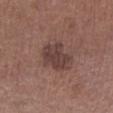Imaged during a routine full-body skin examination; the lesion was not biopsied and no histopathology is available.
Captured under white-light illumination.
This image is a 15 mm lesion crop taken from a total-body photograph.
An algorithmic analysis of the crop reported an area of roughly 10 mm², a shape eccentricity near 0.7, and two-axis asymmetry of about 0.3. The analysis additionally found a lesion color around L≈40 a*≈18 b*≈20 in CIELAB and a normalized border contrast of about 8.
The subject is a male aged 73 to 77.
Located on the left lower leg.